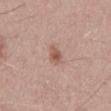Image and clinical context:
A male subject aged approximately 50. Cropped from a total-body skin-imaging series; the visible field is about 15 mm. The recorded lesion diameter is about 2.5 mm. The lesion-visualizer software estimated a color-variation rating of about 3/10 and a peripheral color-asymmetry measure near 1. This is a white-light tile. The lesion is located on the back.